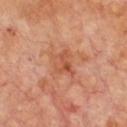From the chest. A lesion tile, about 15 mm wide, cut from a 3D total-body photograph. The tile uses cross-polarized illumination. The lesion's longest dimension is about 3.5 mm. A male subject, aged approximately 70.